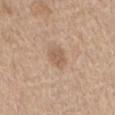This lesion was catalogued during total-body skin photography and was not selected for biopsy. A male subject, approximately 70 years of age. A lesion tile, about 15 mm wide, cut from a 3D total-body photograph. Longest diameter approximately 2.5 mm. Located on the lower back.